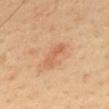From the mid back. Cropped from a total-body skin-imaging series; the visible field is about 15 mm. A male patient, aged around 50.